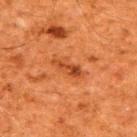Findings:
- follow-up · no biopsy performed (imaged during a skin exam)
- patient · male, aged 58–62
- imaging modality · 15 mm crop, total-body photography
- image-analysis metrics · an average lesion color of about L≈38 a*≈27 b*≈36 (CIELAB) and a lesion–skin lightness drop of about 8; a border-irregularity rating of about 3/10, a color-variation rating of about 4/10, and a peripheral color-asymmetry measure near 1.5; an automated nevus-likeness rating near 5 out of 100 and a detector confidence of about 100 out of 100 that the crop contains a lesion
- diameter · ≈4 mm
- lighting · cross-polarized
- location · the back